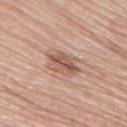notes — imaged on a skin check; not biopsied
image — ~15 mm tile from a whole-body skin photo
subject — female, aged 63–67
body site — the upper back